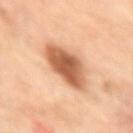Notes:
- workup — catalogued during a skin exam; not biopsied
- size — ≈5.5 mm
- illumination — cross-polarized illumination
- imaging modality — total-body-photography crop, ~15 mm field of view
- site — the right upper arm
- subject — female, aged 63–67